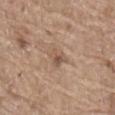This lesion was catalogued during total-body skin photography and was not selected for biopsy. The lesion is on the lower back. The lesion's longest dimension is about 3 mm. A region of skin cropped from a whole-body photographic capture, roughly 15 mm wide. The tile uses white-light illumination. A male patient aged 78–82.